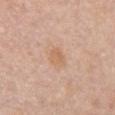| key | value |
|---|---|
| follow-up | imaged on a skin check; not biopsied |
| subject | female, aged approximately 65 |
| image source | total-body-photography crop, ~15 mm field of view |
| TBP lesion metrics | a footprint of about 4.5 mm², an outline eccentricity of about 0.55 (0 = round, 1 = elongated), and a shape-asymmetry score of about 0.3 (0 = symmetric); border irregularity of about 2.5 on a 0–10 scale, internal color variation of about 2 on a 0–10 scale, and radial color variation of about 0.5; an automated nevus-likeness rating near 10 out of 100 and a lesion-detection confidence of about 100/100 |
| size | ≈2.5 mm |
| location | the chest |
| lighting | white-light illumination |